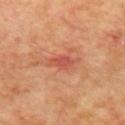Impression: Part of a total-body skin-imaging series; this lesion was reviewed on a skin check and was not flagged for biopsy. Background: From the mid back. The patient is a male roughly 60 years of age. A region of skin cropped from a whole-body photographic capture, roughly 15 mm wide.